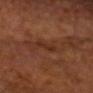• follow-up · total-body-photography surveillance lesion; no biopsy